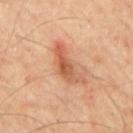<case>
  <biopsy_status>not biopsied; imaged during a skin examination</biopsy_status>
  <image>
    <source>total-body photography crop</source>
    <field_of_view_mm>15</field_of_view_mm>
  </image>
  <automated_metrics>
    <area_mm2_approx>9.0</area_mm2_approx>
    <eccentricity>0.9</eccentricity>
    <shape_asymmetry>0.45</shape_asymmetry>
    <cielab_L>57</cielab_L>
    <cielab_a>25</cielab_a>
    <cielab_b>35</cielab_b>
    <vs_skin_darker_L>11.0</vs_skin_darker_L>
    <vs_skin_contrast_norm>7.5</vs_skin_contrast_norm>
    <nevus_likeness_0_100>65</nevus_likeness_0_100>
    <lesion_detection_confidence_0_100>100</lesion_detection_confidence_0_100>
  </automated_metrics>
  <patient>
    <sex>male</sex>
    <age_approx>65</age_approx>
  </patient>
  <lighting>cross-polarized</lighting>
  <site>mid back</site>
</case>